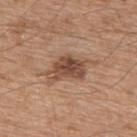Imaged during a routine full-body skin examination; the lesion was not biopsied and no histopathology is available.
Automated image analysis of the tile measured an eccentricity of roughly 0.8.
A male subject, approximately 65 years of age.
A close-up tile cropped from a whole-body skin photograph, about 15 mm across.
About 6 mm across.
On the back.